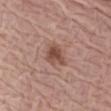Impression:
No biopsy was performed on this lesion — it was imaged during a full skin examination and was not determined to be concerning.
Acquisition and patient details:
Automated image analysis of the tile measured an eccentricity of roughly 0.65 and a shape-asymmetry score of about 0.25 (0 = symmetric). The software also gave a mean CIELAB color near L≈49 a*≈21 b*≈26 and roughly 10 lightness units darker than nearby skin. It also reported a within-lesion color-variation index near 3.5/10 and peripheral color asymmetry of about 1.5. From the arm. A female patient aged 68 to 72. Measured at roughly 4 mm in maximum diameter. The tile uses white-light illumination. This image is a 15 mm lesion crop taken from a total-body photograph.